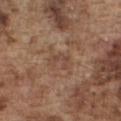| key | value |
|---|---|
| follow-up | catalogued during a skin exam; not biopsied |
| TBP lesion metrics | an area of roughly 3.5 mm² and a shape-asymmetry score of about 0.45 (0 = symmetric); a mean CIELAB color near L≈45 a*≈19 b*≈27, a lesion–skin lightness drop of about 6, and a lesion-to-skin contrast of about 5 (normalized; higher = more distinct); a border-irregularity index near 6/10, a color-variation rating of about 0/10, and a peripheral color-asymmetry measure near 0; a classifier nevus-likeness of about 0/100 and a detector confidence of about 85 out of 100 that the crop contains a lesion |
| image | 15 mm crop, total-body photography |
| illumination | white-light illumination |
| diameter | about 2.5 mm |
| site | the chest |
| subject | male, aged 73 to 77 |